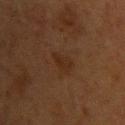workup: catalogued during a skin exam; not biopsied
image-analysis metrics: a lesion area of about 4 mm² and a shape-asymmetry score of about 0.4 (0 = symmetric); a border-irregularity rating of about 3.5/10; lesion-presence confidence of about 100/100
acquisition: total-body-photography crop, ~15 mm field of view
site: the upper back
subject: female, aged around 40
lesion size: ≈3 mm